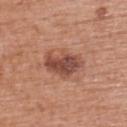biopsy_status: not biopsied; imaged during a skin examination